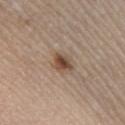automated metrics: an area of roughly 5 mm², a shape eccentricity near 0.6, and a symmetry-axis asymmetry near 0.2; a mean CIELAB color near L≈48 a*≈16 b*≈28, a lesion–skin lightness drop of about 12, and a normalized border contrast of about 9; border irregularity of about 2 on a 0–10 scale, internal color variation of about 4.5 on a 0–10 scale, and peripheral color asymmetry of about 1
image: total-body-photography crop, ~15 mm field of view
anatomic site: the right lower leg
subject: male, aged 43–47
lighting: white-light